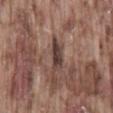Assessment:
The lesion was tiled from a total-body skin photograph and was not biopsied.
Background:
An algorithmic analysis of the crop reported an area of roughly 5 mm² and a shape-asymmetry score of about 0.3 (0 = symmetric). The analysis additionally found a lesion color around L≈39 a*≈16 b*≈20 in CIELAB and a lesion–skin lightness drop of about 13. The analysis additionally found border irregularity of about 3 on a 0–10 scale and peripheral color asymmetry of about 1.5. The analysis additionally found a nevus-likeness score of about 10/100 and lesion-presence confidence of about 70/100. A male patient about 75 years old. A roughly 15 mm field-of-view crop from a total-body skin photograph. The recorded lesion diameter is about 3.5 mm. The tile uses white-light illumination. On the back.